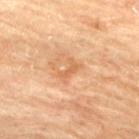notes — no biopsy performed (imaged during a skin exam) | lesion size — ~2.5 mm (longest diameter) | patient — female, approximately 80 years of age | location — the upper back | acquisition — ~15 mm tile from a whole-body skin photo | illumination — cross-polarized.